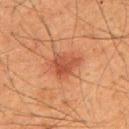follow-up — total-body-photography surveillance lesion; no biopsy
tile lighting — cross-polarized illumination
image-analysis metrics — roughly 8 lightness units darker than nearby skin and a normalized border contrast of about 6.5; a border-irregularity index near 3/10, internal color variation of about 3.5 on a 0–10 scale, and a peripheral color-asymmetry measure near 1; a detector confidence of about 100 out of 100 that the crop contains a lesion
image source — 15 mm crop, total-body photography
size — about 3.5 mm
body site — the upper back
subject — male, aged around 35